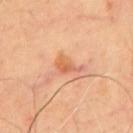Findings:
– notes · total-body-photography surveillance lesion; no biopsy
– patient · male, aged 63–67
– tile lighting · cross-polarized
– diameter · ≈3 mm
– imaging modality · ~15 mm tile from a whole-body skin photo
– automated lesion analysis · a lesion area of about 3.5 mm² and a symmetry-axis asymmetry near 0.35; a lesion–skin lightness drop of about 9 and a lesion-to-skin contrast of about 6.5 (normalized; higher = more distinct); border irregularity of about 3.5 on a 0–10 scale and a color-variation rating of about 3/10; a classifier nevus-likeness of about 25/100 and a detector confidence of about 100 out of 100 that the crop contains a lesion
– body site · the front of the torso